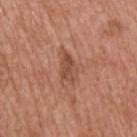  biopsy_status: not biopsied; imaged during a skin examination
  lighting: white-light
  automated_metrics:
    area_mm2_approx: 6.0
    eccentricity: 0.9
    nevus_likeness_0_100: 5
    lesion_detection_confidence_0_100: 100
  lesion_size:
    long_diameter_mm_approx: 4.0
  image:
    source: total-body photography crop
    field_of_view_mm: 15
  patient:
    sex: male
    age_approx: 60
  site: head or neck